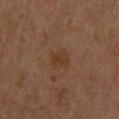No biopsy was performed on this lesion — it was imaged during a full skin examination and was not determined to be concerning. A male patient, aged 48–52. Imaged with cross-polarized lighting. A roughly 15 mm field-of-view crop from a total-body skin photograph. The lesion is located on the upper back.